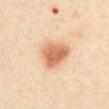Q: Was a biopsy performed?
A: no biopsy performed (imaged during a skin exam)
Q: What kind of image is this?
A: total-body-photography crop, ~15 mm field of view
Q: What are the patient's age and sex?
A: male, roughly 40 years of age
Q: Lesion location?
A: the front of the torso